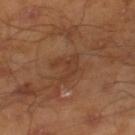notes = no biopsy performed (imaged during a skin exam) | patient = male, roughly 45 years of age | automated lesion analysis = a lesion color around L≈38 a*≈21 b*≈30 in CIELAB, about 6 CIELAB-L* units darker than the surrounding skin, and a normalized border contrast of about 5; border irregularity of about 8 on a 0–10 scale and a within-lesion color-variation index near 1.5/10; a classifier nevus-likeness of about 0/100 and lesion-presence confidence of about 100/100 | location = the leg | lighting = cross-polarized | size = about 3.5 mm | image = total-body-photography crop, ~15 mm field of view.